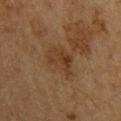Imaged during a routine full-body skin examination; the lesion was not biopsied and no histopathology is available. The patient is a male in their 60s. A 15 mm close-up extracted from a 3D total-body photography capture.